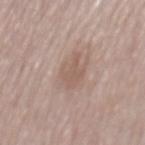Case summary:
- follow-up: total-body-photography surveillance lesion; no biopsy